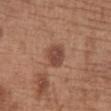Impression:
Part of a total-body skin-imaging series; this lesion was reviewed on a skin check and was not flagged for biopsy.
Acquisition and patient details:
The total-body-photography lesion software estimated an average lesion color of about L≈46 a*≈21 b*≈28 (CIELAB), about 10 CIELAB-L* units darker than the surrounding skin, and a normalized border contrast of about 8. The software also gave a border-irregularity rating of about 1.5/10 and a within-lesion color-variation index near 3.5/10. It also reported a nevus-likeness score of about 75/100 and a detector confidence of about 100 out of 100 that the crop contains a lesion. Measured at roughly 3 mm in maximum diameter. A 15 mm close-up tile from a total-body photography series done for melanoma screening. The lesion is on the chest. The patient is a female about 55 years old. This is a white-light tile.